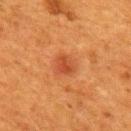Case summary:
• follow-up — catalogued during a skin exam; not biopsied
• patient — female, aged around 40
• lesion diameter — ≈2.5 mm
• tile lighting — cross-polarized illumination
• image — total-body-photography crop, ~15 mm field of view
• location — the upper back
• automated metrics — a mean CIELAB color near L≈41 a*≈28 b*≈36, roughly 8 lightness units darker than nearby skin, and a lesion-to-skin contrast of about 6.5 (normalized; higher = more distinct); border irregularity of about 2.5 on a 0–10 scale and a color-variation rating of about 2.5/10; a lesion-detection confidence of about 100/100